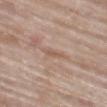  biopsy_status: not biopsied; imaged during a skin examination
  lesion_size:
    long_diameter_mm_approx: 3.0
  image:
    source: total-body photography crop
    field_of_view_mm: 15
  patient:
    sex: male
    age_approx: 80
  site: left thigh
  lighting: white-light
  automated_metrics:
    cielab_L: 57
    cielab_a: 17
    cielab_b: 27
    vs_skin_darker_L: 7.0
    vs_skin_contrast_norm: 5.0
    border_irregularity_0_10: 2.0
    color_variation_0_10: 0.0
    peripheral_color_asymmetry: 0.0
    nevus_likeness_0_100: 0
    lesion_detection_confidence_0_100: 70